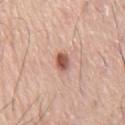Assessment: This lesion was catalogued during total-body skin photography and was not selected for biopsy. Acquisition and patient details: A close-up tile cropped from a whole-body skin photograph, about 15 mm across. A male patient, in their mid- to late 70s. The lesion is located on the chest. Approximately 2.5 mm at its widest.